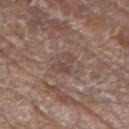notes: catalogued during a skin exam; not biopsied | image-analysis metrics: a lesion color around L≈45 a*≈16 b*≈22 in CIELAB, about 7 CIELAB-L* units darker than the surrounding skin, and a lesion-to-skin contrast of about 5.5 (normalized; higher = more distinct) | lesion size: ~3 mm (longest diameter) | anatomic site: the left forearm | tile lighting: white-light illumination | image source: total-body-photography crop, ~15 mm field of view | patient: male, in their 80s.